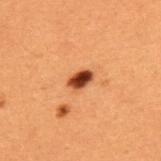notes: imaged on a skin check; not biopsied
body site: the upper back
imaging modality: ~15 mm tile from a whole-body skin photo
subject: male, aged around 50